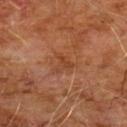biopsy status = total-body-photography surveillance lesion; no biopsy
subject = male, roughly 60 years of age
image source = total-body-photography crop, ~15 mm field of view
lesion diameter = about 2.5 mm
automated lesion analysis = a classifier nevus-likeness of about 0/100 and a detector confidence of about 100 out of 100 that the crop contains a lesion
location = the chest